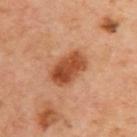Case summary:
* workup: imaged on a skin check; not biopsied
* subject: male, roughly 65 years of age
* image source: 15 mm crop, total-body photography
* body site: the upper back